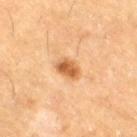Part of a total-body skin-imaging series; this lesion was reviewed on a skin check and was not flagged for biopsy. Cropped from a whole-body photographic skin survey; the tile spans about 15 mm. About 3 mm across. The total-body-photography lesion software estimated a nevus-likeness score of about 90/100 and a lesion-detection confidence of about 100/100. The patient is a male approximately 60 years of age. From the right thigh.